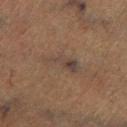Assessment: Part of a total-body skin-imaging series; this lesion was reviewed on a skin check and was not flagged for biopsy. Background: Automated image analysis of the tile measured a footprint of about 6.5 mm². The software also gave a classifier nevus-likeness of about 0/100 and lesion-presence confidence of about 70/100. A male subject, aged 73 to 77. From the leg. Approximately 5 mm at its widest. Captured under cross-polarized illumination. A 15 mm close-up tile from a total-body photography series done for melanoma screening.